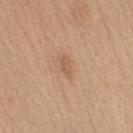Case summary:
- biopsy status — no biopsy performed (imaged during a skin exam)
- automated lesion analysis — a footprint of about 2.5 mm², an eccentricity of roughly 0.9, and two-axis asymmetry of about 0.3; an average lesion color of about L≈58 a*≈20 b*≈34 (CIELAB) and a lesion-to-skin contrast of about 5 (normalized; higher = more distinct); a classifier nevus-likeness of about 5/100
- site — the arm
- illumination — white-light
- imaging modality — ~15 mm tile from a whole-body skin photo
- patient — male, aged around 70